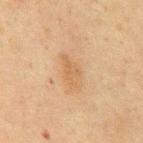Assessment: Captured during whole-body skin photography for melanoma surveillance; the lesion was not biopsied.